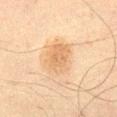notes — no biopsy performed (imaged during a skin exam) | site — the leg | tile lighting — cross-polarized | image source — total-body-photography crop, ~15 mm field of view | subject — male, roughly 60 years of age | lesion size — ~4 mm (longest diameter).